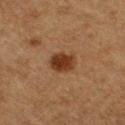biopsy status = no biopsy performed (imaged during a skin exam)
image = ~15 mm crop, total-body skin-cancer survey
illumination = cross-polarized illumination
subject = male, roughly 75 years of age
diameter = about 3 mm
location = the right thigh